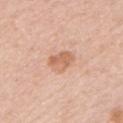Clinical impression: No biopsy was performed on this lesion — it was imaged during a full skin examination and was not determined to be concerning. Background: The patient is a female in their mid-40s. This is a white-light tile. Longest diameter approximately 3.5 mm. A region of skin cropped from a whole-body photographic capture, roughly 15 mm wide. From the left upper arm. Automated tile analysis of the lesion measured a footprint of about 7 mm², an eccentricity of roughly 0.7, and a symmetry-axis asymmetry near 0.2. And it measured an average lesion color of about L≈64 a*≈22 b*≈33 (CIELAB), about 10 CIELAB-L* units darker than the surrounding skin, and a lesion-to-skin contrast of about 6.5 (normalized; higher = more distinct). And it measured border irregularity of about 2 on a 0–10 scale. It also reported an automated nevus-likeness rating near 10 out of 100.